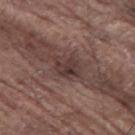Assessment: Recorded during total-body skin imaging; not selected for excision or biopsy. Background: Automated tile analysis of the lesion measured a classifier nevus-likeness of about 0/100. A 15 mm close-up tile from a total-body photography series done for melanoma screening. Imaged with white-light lighting. From the left thigh. The patient is a male aged 78–82.